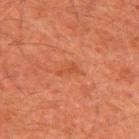Q: Where on the body is the lesion?
A: the upper back
Q: What are the patient's age and sex?
A: male, aged around 60
Q: How was this image acquired?
A: total-body-photography crop, ~15 mm field of view
Q: What lighting was used for the tile?
A: cross-polarized illumination
Q: What is the lesion's diameter?
A: about 3 mm